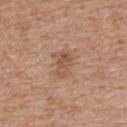Clinical impression:
Imaged during a routine full-body skin examination; the lesion was not biopsied and no histopathology is available.
Background:
A female subject, aged 68–72. The lesion's longest dimension is about 3 mm. Located on the back. An algorithmic analysis of the crop reported a footprint of about 6 mm², a shape eccentricity near 0.7, and a shape-asymmetry score of about 0.35 (0 = symmetric). The software also gave a border-irregularity rating of about 4/10 and internal color variation of about 3.5 on a 0–10 scale. It also reported an automated nevus-likeness rating near 5 out of 100. This image is a 15 mm lesion crop taken from a total-body photograph. Imaged with white-light lighting.